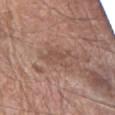<case>
  <biopsy_status>not biopsied; imaged during a skin examination</biopsy_status>
  <image>
    <source>total-body photography crop</source>
    <field_of_view_mm>15</field_of_view_mm>
  </image>
  <patient>
    <sex>male</sex>
    <age_approx>70</age_approx>
  </patient>
  <site>arm</site>
  <lesion_size>
    <long_diameter_mm_approx>3.5</long_diameter_mm_approx>
  </lesion_size>
</case>